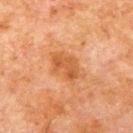Q: What is the imaging modality?
A: ~15 mm tile from a whole-body skin photo
Q: What are the patient's age and sex?
A: male, approximately 80 years of age
Q: Where on the body is the lesion?
A: the left upper arm